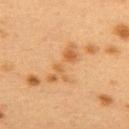| key | value |
|---|---|
| workup | catalogued during a skin exam; not biopsied |
| anatomic site | the upper back |
| size | ≈5.5 mm |
| image | ~15 mm tile from a whole-body skin photo |
| patient | female, about 40 years old |
| automated metrics | an area of roughly 8.5 mm² and a symmetry-axis asymmetry near 0.45; a lesion–skin lightness drop of about 7 and a normalized border contrast of about 6 |
| illumination | cross-polarized illumination |